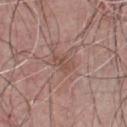{"biopsy_status": "not biopsied; imaged during a skin examination", "site": "chest", "patient": {"sex": "male", "age_approx": 65}, "lighting": "white-light", "image": {"source": "total-body photography crop", "field_of_view_mm": 15}}